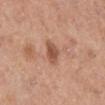workup: catalogued during a skin exam; not biopsied
subject: female, roughly 40 years of age
illumination: white-light
imaging modality: 15 mm crop, total-body photography
lesion size: about 3 mm
body site: the left lower leg